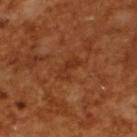biopsy status: catalogued during a skin exam; not biopsied
subject: male, approximately 65 years of age
imaging modality: 15 mm crop, total-body photography
lesion diameter: ~3 mm (longest diameter)
tile lighting: cross-polarized illumination